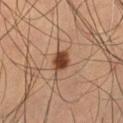biopsy status — catalogued during a skin exam; not biopsied
site — the leg
image source — ~15 mm crop, total-body skin-cancer survey
patient — male, aged 48–52
lesion diameter — ~2.5 mm (longest diameter)